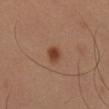Captured during whole-body skin photography for melanoma surveillance; the lesion was not biopsied.
Cropped from a total-body skin-imaging series; the visible field is about 15 mm.
The lesion is located on the right thigh.
The tile uses cross-polarized illumination.
A male patient aged around 50.
Measured at roughly 2.5 mm in maximum diameter.